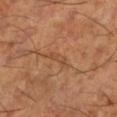Assessment: Recorded during total-body skin imaging; not selected for excision or biopsy. Image and clinical context: The lesion's longest dimension is about 2.5 mm. The lesion-visualizer software estimated a nevus-likeness score of about 0/100 and a lesion-detection confidence of about 70/100. The patient is a male about 65 years old. Located on the left lower leg. This is a cross-polarized tile. Cropped from a total-body skin-imaging series; the visible field is about 15 mm.